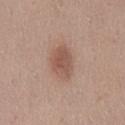| field | value |
|---|---|
| follow-up | no biopsy performed (imaged during a skin exam) |
| patient | female, aged around 40 |
| imaging modality | ~15 mm crop, total-body skin-cancer survey |
| site | the mid back |